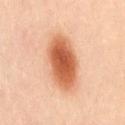follow-up: catalogued during a skin exam; not biopsied | illumination: cross-polarized | size: ≈6.5 mm | site: the right thigh | subject: female, roughly 40 years of age | imaging modality: 15 mm crop, total-body photography.